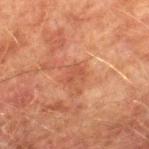The lesion was photographed on a routine skin check and not biopsied; there is no pathology result.
About 2.5 mm across.
From the right lower leg.
A 15 mm close-up extracted from a 3D total-body photography capture.
A male patient, aged around 75.
Automated tile analysis of the lesion measured an area of roughly 4 mm² and a symmetry-axis asymmetry near 0.35. The software also gave a lesion color around L≈44 a*≈24 b*≈30 in CIELAB and a normalized border contrast of about 5.